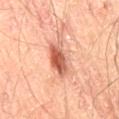biopsy status: imaged on a skin check; not biopsied
tile lighting: cross-polarized
patient: male, approximately 65 years of age
site: the right thigh
imaging modality: 15 mm crop, total-body photography
diameter: about 4.5 mm